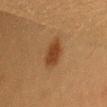Q: Is there a histopathology result?
A: imaged on a skin check; not biopsied
Q: Automated lesion metrics?
A: an area of roughly 7 mm² and an eccentricity of roughly 0.7; a border-irregularity rating of about 2/10, a within-lesion color-variation index near 2/10, and radial color variation of about 0.5
Q: How large is the lesion?
A: about 3.5 mm
Q: What is the imaging modality?
A: 15 mm crop, total-body photography
Q: Who is the patient?
A: female, about 40 years old
Q: How was the tile lit?
A: cross-polarized
Q: Lesion location?
A: the right thigh